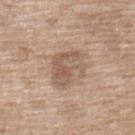Imaged during a routine full-body skin examination; the lesion was not biopsied and no histopathology is available. Located on the upper back. A female patient aged 73 to 77. Captured under white-light illumination. A 15 mm close-up tile from a total-body photography series done for melanoma screening. Automated image analysis of the tile measured an average lesion color of about L≈56 a*≈17 b*≈27 (CIELAB). Measured at roughly 4.5 mm in maximum diameter.